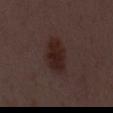<case>
  <biopsy_status>not biopsied; imaged during a skin examination</biopsy_status>
  <automated_metrics>
    <area_mm2_approx>12.0</area_mm2_approx>
    <eccentricity>0.9</eccentricity>
    <shape_asymmetry>0.15</shape_asymmetry>
    <cielab_L>23</cielab_L>
    <cielab_a>16</cielab_a>
    <cielab_b>17</cielab_b>
    <vs_skin_darker_L>7.0</vs_skin_darker_L>
    <vs_skin_contrast_norm>9.0</vs_skin_contrast_norm>
    <nevus_likeness_0_100>85</nevus_likeness_0_100>
    <lesion_detection_confidence_0_100>100</lesion_detection_confidence_0_100>
  </automated_metrics>
  <image>
    <source>total-body photography crop</source>
    <field_of_view_mm>15</field_of_view_mm>
  </image>
  <patient>
    <sex>male</sex>
    <age_approx>50</age_approx>
  </patient>
  <lesion_size>
    <long_diameter_mm_approx>5.5</long_diameter_mm_approx>
  </lesion_size>
  <lighting>white-light</lighting>
</case>